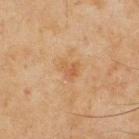notes: imaged on a skin check; not biopsied | tile lighting: cross-polarized illumination | lesion diameter: ~2.5 mm (longest diameter) | image: 15 mm crop, total-body photography | automated lesion analysis: an area of roughly 4.5 mm², an eccentricity of roughly 0.6, and a symmetry-axis asymmetry near 0.35; internal color variation of about 3 on a 0–10 scale and radial color variation of about 1 | body site: the upper back | patient: male, aged around 45.